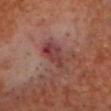  biopsy_status: not biopsied; imaged during a skin examination
  site: head or neck
  lesion_size:
    long_diameter_mm_approx: 5.0
  patient:
    sex: male
    age_approx: 60
  automated_metrics:
    cielab_L: 39
    cielab_a: 24
    cielab_b: 22
    vs_skin_darker_L: 8.0
    vs_skin_contrast_norm: 7.0
    border_irregularity_0_10: 4.5
    color_variation_0_10: 5.5
  image:
    source: total-body photography crop
    field_of_view_mm: 15